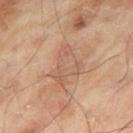No biopsy was performed on this lesion — it was imaged during a full skin examination and was not determined to be concerning. Measured at roughly 5 mm in maximum diameter. A region of skin cropped from a whole-body photographic capture, roughly 15 mm wide. A male patient, aged 63–67. On the right thigh. The total-body-photography lesion software estimated a lesion color around L≈59 a*≈20 b*≈31 in CIELAB, a lesion–skin lightness drop of about 7, and a lesion-to-skin contrast of about 4.5 (normalized; higher = more distinct). The analysis additionally found a detector confidence of about 100 out of 100 that the crop contains a lesion. The tile uses cross-polarized illumination.